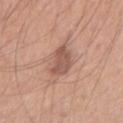| field | value |
|---|---|
| workup | total-body-photography surveillance lesion; no biopsy |
| lighting | white-light illumination |
| imaging modality | ~15 mm tile from a whole-body skin photo |
| size | about 4.5 mm |
| patient | male, aged 28–32 |
| TBP lesion metrics | a lesion area of about 7.5 mm², a shape eccentricity near 0.9, and a symmetry-axis asymmetry near 0.2; border irregularity of about 3 on a 0–10 scale and a within-lesion color-variation index near 2/10 |
| site | the right upper arm |